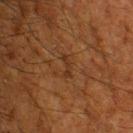{
  "biopsy_status": "not biopsied; imaged during a skin examination",
  "site": "head or neck",
  "patient": {
    "sex": "male",
    "age_approx": 60
  },
  "image": {
    "source": "total-body photography crop",
    "field_of_view_mm": 15
  }
}